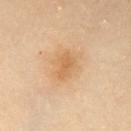Findings:
• follow-up — total-body-photography surveillance lesion; no biopsy
• automated metrics — an area of roughly 6.5 mm² and two-axis asymmetry of about 0.25; an average lesion color of about L≈66 a*≈20 b*≈41 (CIELAB), a lesion–skin lightness drop of about 8, and a normalized border contrast of about 6; a classifier nevus-likeness of about 20/100 and a detector confidence of about 100 out of 100 that the crop contains a lesion
• imaging modality — 15 mm crop, total-body photography
• location — the front of the torso
• lesion size — ~3.5 mm (longest diameter)
• lighting — cross-polarized illumination
• patient — female, roughly 40 years of age